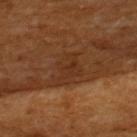Clinical impression: Recorded during total-body skin imaging; not selected for excision or biopsy. Clinical summary: About 4 mm across. The lesion-visualizer software estimated a normalized border contrast of about 6. The analysis additionally found internal color variation of about 2.5 on a 0–10 scale and a peripheral color-asymmetry measure near 1. A male subject roughly 65 years of age. From the upper back. Cropped from a whole-body photographic skin survey; the tile spans about 15 mm. Captured under cross-polarized illumination.